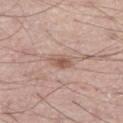follow-up: imaged on a skin check; not biopsied | size: ≈2.5 mm | patient: male, in their 60s | acquisition: 15 mm crop, total-body photography | site: the right thigh | automated lesion analysis: an area of roughly 4 mm².